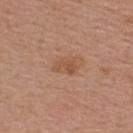Notes:
– follow-up · total-body-photography surveillance lesion; no biopsy
– lesion diameter · about 3 mm
– subject · male, aged around 55
– acquisition · ~15 mm tile from a whole-body skin photo
– image-analysis metrics · a lesion color around L≈52 a*≈21 b*≈33 in CIELAB and roughly 7 lightness units darker than nearby skin; a border-irregularity rating of about 3.5/10, internal color variation of about 2 on a 0–10 scale, and a peripheral color-asymmetry measure near 0.5; a classifier nevus-likeness of about 5/100 and a detector confidence of about 100 out of 100 that the crop contains a lesion
– tile lighting · white-light illumination
– site · the upper back